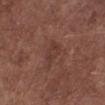The lesion was photographed on a routine skin check and not biopsied; there is no pathology result.
A male patient, in their mid- to late 50s.
A close-up tile cropped from a whole-body skin photograph, about 15 mm across.
This is a white-light tile.
The lesion's longest dimension is about 3 mm.
The lesion is on the left lower leg.